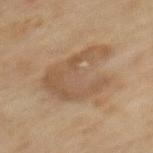{"biopsy_status": "not biopsied; imaged during a skin examination", "lesion_size": {"long_diameter_mm_approx": 7.5}, "site": "mid back", "automated_metrics": {"cielab_L": 46, "cielab_a": 14, "cielab_b": 28, "vs_skin_darker_L": 7.0, "vs_skin_contrast_norm": 6.0, "nevus_likeness_0_100": 0}, "lighting": "cross-polarized", "patient": {"sex": "female", "age_approx": 60}, "image": {"source": "total-body photography crop", "field_of_view_mm": 15}}